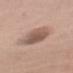Clinical impression:
The lesion was tiled from a total-body skin photograph and was not biopsied.
Background:
A male subject, aged approximately 60. A close-up tile cropped from a whole-body skin photograph, about 15 mm across. On the left upper arm.